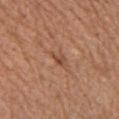workup = no biopsy performed (imaged during a skin exam); subject = female, aged 53 to 57; site = the chest; acquisition = ~15 mm crop, total-body skin-cancer survey.